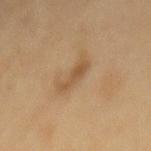site = the mid back | imaging modality = ~15 mm tile from a whole-body skin photo | subject = female, in their mid- to late 50s | illumination = cross-polarized illumination | size = ≈3.5 mm.